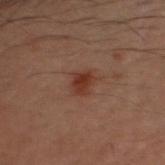{"biopsy_status": "not biopsied; imaged during a skin examination", "automated_metrics": {"eccentricity": 0.7, "shape_asymmetry": 0.3, "cielab_L": 27, "cielab_a": 19, "cielab_b": 23, "vs_skin_darker_L": 7.0, "vs_skin_contrast_norm": 8.5, "border_irregularity_0_10": 3.0, "color_variation_0_10": 2.5, "peripheral_color_asymmetry": 1.0, "nevus_likeness_0_100": 90, "lesion_detection_confidence_0_100": 100}, "lighting": "cross-polarized", "site": "head or neck", "image": {"source": "total-body photography crop", "field_of_view_mm": 15}, "lesion_size": {"long_diameter_mm_approx": 2.5}, "patient": {"sex": "male", "age_approx": 30}}